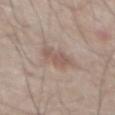Findings:
- lesion size — about 3.5 mm
- anatomic site — the mid back
- illumination — white-light
- image source — total-body-photography crop, ~15 mm field of view
- automated lesion analysis — a classifier nevus-likeness of about 60/100 and lesion-presence confidence of about 100/100
- subject — male, in their mid-50s